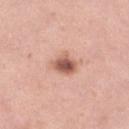Notes:
* notes — no biopsy performed (imaged during a skin exam)
* subject — female, aged approximately 50
* anatomic site — the left lower leg
* imaging modality — 15 mm crop, total-body photography